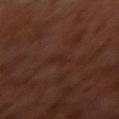image:
  source: total-body photography crop
  field_of_view_mm: 15
patient:
  sex: male
  age_approx: 30
lighting: cross-polarized
site: left arm